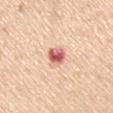follow-up: no biopsy performed (imaged during a skin exam) | image source: total-body-photography crop, ~15 mm field of view | body site: the arm | automated metrics: an average lesion color of about L≈63 a*≈31 b*≈27 (CIELAB), a lesion–skin lightness drop of about 18, and a normalized border contrast of about 11; border irregularity of about 1.5 on a 0–10 scale, internal color variation of about 6.5 on a 0–10 scale, and a peripheral color-asymmetry measure near 2; a nevus-likeness score of about 0/100 | diameter: ≈3 mm | patient: male, aged 58–62.